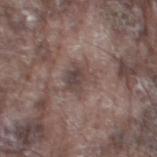The lesion was tiled from a total-body skin photograph and was not biopsied. Automated tile analysis of the lesion measured a classifier nevus-likeness of about 0/100 and lesion-presence confidence of about 95/100. The lesion is located on the left thigh. A male subject, aged around 75. A 15 mm crop from a total-body photograph taken for skin-cancer surveillance. Measured at roughly 3.5 mm in maximum diameter.